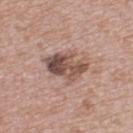Case summary:
• subject — female, in their 40s
• diameter — about 5.5 mm
• lighting — white-light illumination
• imaging modality — ~15 mm tile from a whole-body skin photo
• body site — the upper back
• automated metrics — an average lesion color of about L≈51 a*≈18 b*≈24 (CIELAB) and a lesion-to-skin contrast of about 9 (normalized; higher = more distinct); a border-irregularity rating of about 3.5/10, a within-lesion color-variation index near 9/10, and a peripheral color-asymmetry measure near 3; an automated nevus-likeness rating near 45 out of 100 and lesion-presence confidence of about 100/100